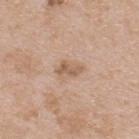workup: catalogued during a skin exam; not biopsied | anatomic site: the upper back | acquisition: total-body-photography crop, ~15 mm field of view | subject: male, in their mid- to late 60s.